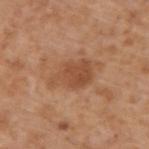Captured during whole-body skin photography for melanoma surveillance; the lesion was not biopsied.